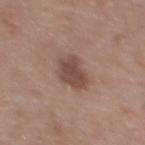Findings:
- workup: imaged on a skin check; not biopsied
- body site: the upper back
- imaging modality: total-body-photography crop, ~15 mm field of view
- tile lighting: white-light illumination
- lesion size: ≈3.5 mm
- automated lesion analysis: an area of roughly 9 mm² and a shape eccentricity near 0.45; a mean CIELAB color near L≈47 a*≈18 b*≈24, about 11 CIELAB-L* units darker than the surrounding skin, and a lesion-to-skin contrast of about 8 (normalized; higher = more distinct)
- patient: female, in their 50s